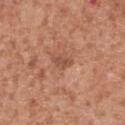This lesion was catalogued during total-body skin photography and was not selected for biopsy.
From the chest.
A male patient aged 63 to 67.
A roughly 15 mm field-of-view crop from a total-body skin photograph.